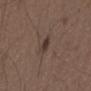biopsy status=catalogued during a skin exam; not biopsied | subject=male, aged 48 to 52 | imaging modality=15 mm crop, total-body photography | site=the chest.